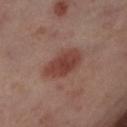| field | value |
|---|---|
| follow-up | no biopsy performed (imaged during a skin exam) |
| illumination | cross-polarized illumination |
| acquisition | ~15 mm crop, total-body skin-cancer survey |
| patient | female, aged 53–57 |
| site | the left lower leg |